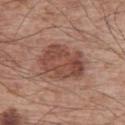workup — no biopsy performed (imaged during a skin exam)
lesion size — ≈6 mm
acquisition — ~15 mm crop, total-body skin-cancer survey
lighting — white-light
patient — male, roughly 65 years of age
site — the right upper arm